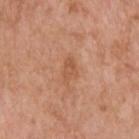The patient is a male approximately 60 years of age. Cropped from a total-body skin-imaging series; the visible field is about 15 mm. Located on the back.